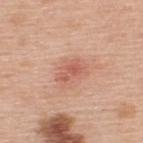<case>
  <biopsy_status>not biopsied; imaged during a skin examination</biopsy_status>
  <lesion_size>
    <long_diameter_mm_approx>3.0</long_diameter_mm_approx>
  </lesion_size>
  <patient>
    <sex>male</sex>
    <age_approx>50</age_approx>
  </patient>
  <site>upper back</site>
  <image>
    <source>total-body photography crop</source>
    <field_of_view_mm>15</field_of_view_mm>
  </image>
</case>